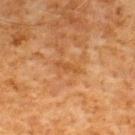Case summary:
* follow-up: no biopsy performed (imaged during a skin exam)